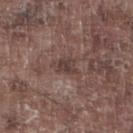No biopsy was performed on this lesion — it was imaged during a full skin examination and was not determined to be concerning. On the right lower leg. A male subject, aged 73 to 77. This is a white-light tile. The lesion's longest dimension is about 3 mm. A 15 mm close-up tile from a total-body photography series done for melanoma screening.